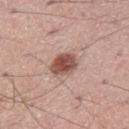  biopsy_status: not biopsied; imaged during a skin examination
  lighting: white-light
  patient:
    sex: male
    age_approx: 55
  image:
    source: total-body photography crop
    field_of_view_mm: 15
  lesion_size:
    long_diameter_mm_approx: 3.5